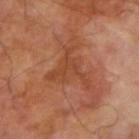notes: catalogued during a skin exam; not biopsied | imaging modality: ~15 mm tile from a whole-body skin photo | location: the arm | subject: male, about 70 years old.